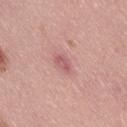Automated tile analysis of the lesion measured a footprint of about 3 mm², a shape eccentricity near 0.85, and a shape-asymmetry score of about 0.3 (0 = symmetric). The analysis additionally found a mean CIELAB color near L≈57 a*≈27 b*≈20, a lesion–skin lightness drop of about 9, and a normalized border contrast of about 6.5. The analysis additionally found a color-variation rating of about 2/10. The analysis additionally found a nevus-likeness score of about 0/100.
This is a white-light tile.
From the leg.
A male subject, aged approximately 40.
A region of skin cropped from a whole-body photographic capture, roughly 15 mm wide.
Approximately 2.5 mm at its widest.